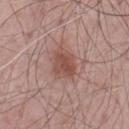Part of a total-body skin-imaging series; this lesion was reviewed on a skin check and was not flagged for biopsy. From the chest. A male subject roughly 65 years of age. Captured under white-light illumination. This image is a 15 mm lesion crop taken from a total-body photograph. The recorded lesion diameter is about 3.5 mm.